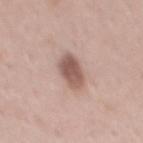Q: How was this image acquired?
A: ~15 mm tile from a whole-body skin photo
Q: Who is the patient?
A: male, aged around 45
Q: Lesion location?
A: the mid back